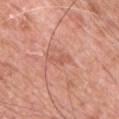Q: Is there a histopathology result?
A: catalogued during a skin exam; not biopsied
Q: Automated lesion metrics?
A: an outline eccentricity of about 0.95 (0 = round, 1 = elongated) and a shape-asymmetry score of about 0.35 (0 = symmetric); a mean CIELAB color near L≈58 a*≈27 b*≈30, about 7 CIELAB-L* units darker than the surrounding skin, and a normalized border contrast of about 5; a border-irregularity index near 4.5/10, a within-lesion color-variation index near 0/10, and a peripheral color-asymmetry measure near 0
Q: Patient demographics?
A: male, aged around 60
Q: What is the imaging modality?
A: total-body-photography crop, ~15 mm field of view
Q: Where on the body is the lesion?
A: the chest
Q: Lesion size?
A: ≈3 mm